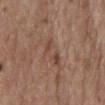| key | value |
|---|---|
| follow-up | total-body-photography surveillance lesion; no biopsy |
| patient | female, aged 63–67 |
| TBP lesion metrics | a detector confidence of about 50 out of 100 that the crop contains a lesion |
| tile lighting | white-light |
| location | the mid back |
| acquisition | total-body-photography crop, ~15 mm field of view |